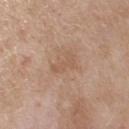workup: catalogued during a skin exam; not biopsied
imaging modality: ~15 mm tile from a whole-body skin photo
subject: female, aged around 55
size: ≈3 mm
TBP lesion metrics: an area of roughly 4 mm², a shape eccentricity near 0.8, and a shape-asymmetry score of about 0.45 (0 = symmetric); a nevus-likeness score of about 0/100 and a lesion-detection confidence of about 100/100
anatomic site: the right upper arm